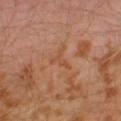This lesion was catalogued during total-body skin photography and was not selected for biopsy. The total-body-photography lesion software estimated an eccentricity of roughly 0.8. The analysis additionally found internal color variation of about 0 on a 0–10 scale and radial color variation of about 0. Located on the left leg. A 15 mm close-up tile from a total-body photography series done for melanoma screening. A male subject, aged 28–32. This is a cross-polarized tile.